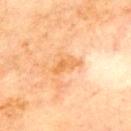Findings:
- biopsy status · catalogued during a skin exam; not biopsied
- patient · male, in their 70s
- location · the front of the torso
- image source · total-body-photography crop, ~15 mm field of view
- diameter · ~4 mm (longest diameter)
- illumination · cross-polarized